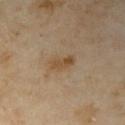The lesion was photographed on a routine skin check and not biopsied; there is no pathology result.
Cropped from a total-body skin-imaging series; the visible field is about 15 mm.
The subject is a female aged 33–37.
From the right upper arm.